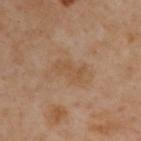  biopsy_status: not biopsied; imaged during a skin examination
  lighting: cross-polarized
  automated_metrics:
    area_mm2_approx: 6.5
    eccentricity: 0.85
    shape_asymmetry: 0.35
    border_irregularity_0_10: 4.0
    color_variation_0_10: 1.0
    nevus_likeness_0_100: 0
  image:
    source: total-body photography crop
    field_of_view_mm: 15
  patient:
    sex: male
    age_approx: 55
  site: back
  lesion_size:
    long_diameter_mm_approx: 4.0